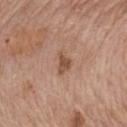No biopsy was performed on this lesion — it was imaged during a full skin examination and was not determined to be concerning. Cropped from a whole-body photographic skin survey; the tile spans about 15 mm. A female patient aged approximately 60. The lesion is on the chest. Imaged with white-light lighting.